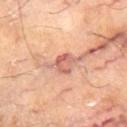The lesion was tiled from a total-body skin photograph and was not biopsied.